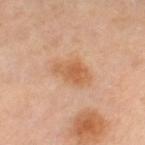The lesion was photographed on a routine skin check and not biopsied; there is no pathology result. A 15 mm close-up extracted from a 3D total-body photography capture. Approximately 4 mm at its widest. Captured under cross-polarized illumination. On the leg. A female subject in their 50s. Automated tile analysis of the lesion measured a footprint of about 8 mm², a shape eccentricity near 0.8, and two-axis asymmetry of about 0.2.